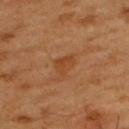notes = total-body-photography surveillance lesion; no biopsy | site = the upper back | subject = male, aged around 60 | acquisition = total-body-photography crop, ~15 mm field of view | automated metrics = a lesion color around L≈43 a*≈24 b*≈37 in CIELAB, roughly 6 lightness units darker than nearby skin, and a normalized lesion–skin contrast near 6; a nevus-likeness score of about 20/100 and a lesion-detection confidence of about 100/100 | tile lighting = cross-polarized illumination.